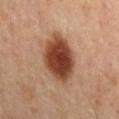Captured during whole-body skin photography for melanoma surveillance; the lesion was not biopsied. An algorithmic analysis of the crop reported an area of roughly 19 mm² and a shape-asymmetry score of about 0.1 (0 = symmetric). It also reported a color-variation rating of about 5.5/10 and radial color variation of about 1.5. And it measured an automated nevus-likeness rating near 100 out of 100 and a lesion-detection confidence of about 100/100. Measured at roughly 6.5 mm in maximum diameter. A 15 mm crop from a total-body photograph taken for skin-cancer surveillance. On the back. Captured under cross-polarized illumination. A male subject aged approximately 55.